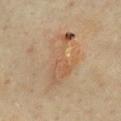| feature | finding |
|---|---|
| follow-up | total-body-photography surveillance lesion; no biopsy |
| anatomic site | the chest |
| lesion diameter | about 7.5 mm |
| lighting | cross-polarized illumination |
| acquisition | ~15 mm tile from a whole-body skin photo |
| image-analysis metrics | an eccentricity of roughly 0.9 and a shape-asymmetry score of about 0.5 (0 = symmetric); roughly 7 lightness units darker than nearby skin and a normalized lesion–skin contrast near 5.5; a border-irregularity index near 7/10, a color-variation rating of about 5.5/10, and radial color variation of about 2; a detector confidence of about 95 out of 100 that the crop contains a lesion |
| subject | female, aged around 60 |